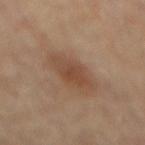notes: no biopsy performed (imaged during a skin exam); subject: male, aged around 85 or older; body site: the mid back; image: total-body-photography crop, ~15 mm field of view.